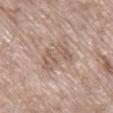workup: total-body-photography surveillance lesion; no biopsy
lesion diameter: ≈5 mm
illumination: white-light
acquisition: total-body-photography crop, ~15 mm field of view
location: the right lower leg
TBP lesion metrics: a border-irregularity rating of about 5/10 and internal color variation of about 3 on a 0–10 scale; a classifier nevus-likeness of about 0/100 and lesion-presence confidence of about 75/100
patient: female, approximately 75 years of age